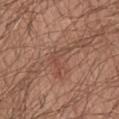biopsy status = imaged on a skin check; not biopsied | lesion diameter = ~4 mm (longest diameter) | image-analysis metrics = a nevus-likeness score of about 0/100 | lighting = white-light | subject = male, aged approximately 65 | location = the chest | image source = ~15 mm crop, total-body skin-cancer survey.